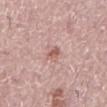Q: Was this lesion biopsied?
A: no biopsy performed (imaged during a skin exam)
Q: What is the anatomic site?
A: the mid back
Q: Illumination type?
A: white-light
Q: What is the imaging modality?
A: total-body-photography crop, ~15 mm field of view
Q: Who is the patient?
A: male, aged approximately 75
Q: Lesion size?
A: ~3 mm (longest diameter)